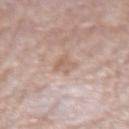notes — no biopsy performed (imaged during a skin exam) | image source — ~15 mm crop, total-body skin-cancer survey | patient — female, in their 70s | anatomic site — the right forearm.